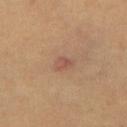notes: no biopsy performed (imaged during a skin exam)
patient: male, aged around 60
location: the front of the torso
acquisition: ~15 mm crop, total-body skin-cancer survey
illumination: cross-polarized illumination
automated lesion analysis: a lesion area of about 3 mm², a shape eccentricity near 0.75, and a symmetry-axis asymmetry near 0.4; a mean CIELAB color near L≈49 a*≈20 b*≈26, a lesion–skin lightness drop of about 7, and a lesion-to-skin contrast of about 6 (normalized; higher = more distinct)
diameter: ~2.5 mm (longest diameter)